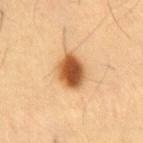Q: Was this lesion biopsied?
A: total-body-photography surveillance lesion; no biopsy
Q: What kind of image is this?
A: 15 mm crop, total-body photography
Q: Who is the patient?
A: male, aged approximately 55
Q: Where on the body is the lesion?
A: the front of the torso
Q: Illumination type?
A: cross-polarized illumination
Q: What is the lesion's diameter?
A: ≈4 mm